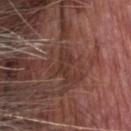follow-up: imaged on a skin check; not biopsied
body site: the head or neck
image source: ~15 mm tile from a whole-body skin photo
TBP lesion metrics: a footprint of about 5 mm², an eccentricity of roughly 0.85, and a shape-asymmetry score of about 0.5 (0 = symmetric); a mean CIELAB color near L≈34 a*≈20 b*≈23 and a normalized border contrast of about 6.5
tile lighting: white-light
diameter: ~4 mm (longest diameter)
patient: male, approximately 75 years of age